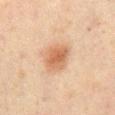  biopsy_status: not biopsied; imaged during a skin examination
  patient:
    sex: male
    age_approx: 70
  image:
    source: total-body photography crop
    field_of_view_mm: 15
  site: back
  automated_metrics:
    nevus_likeness_0_100: 100
    lesion_detection_confidence_0_100: 100
  lighting: cross-polarized
  lesion_size:
    long_diameter_mm_approx: 3.5